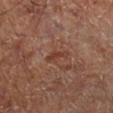Q: Was this lesion biopsied?
A: imaged on a skin check; not biopsied
Q: Automated lesion metrics?
A: a lesion area of about 3 mm², a shape eccentricity near 0.9, and a shape-asymmetry score of about 0.35 (0 = symmetric); an average lesion color of about L≈38 a*≈22 b*≈28 (CIELAB) and a normalized border contrast of about 6.5; a border-irregularity rating of about 4/10, internal color variation of about 0.5 on a 0–10 scale, and a peripheral color-asymmetry measure near 0
Q: Where on the body is the lesion?
A: the right lower leg
Q: What lighting was used for the tile?
A: cross-polarized illumination
Q: Who is the patient?
A: aged approximately 65
Q: How was this image acquired?
A: 15 mm crop, total-body photography